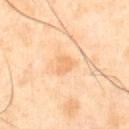notes — imaged on a skin check; not biopsied | site — the abdomen | patient — male, roughly 50 years of age | acquisition — total-body-photography crop, ~15 mm field of view.